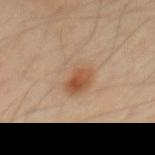The lesion was tiled from a total-body skin photograph and was not biopsied. A lesion tile, about 15 mm wide, cut from a 3D total-body photograph. The patient is a male aged around 50. The lesion's longest dimension is about 4 mm. Captured under cross-polarized illumination. The lesion is located on the mid back.